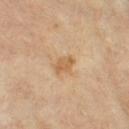Clinical impression: Part of a total-body skin-imaging series; this lesion was reviewed on a skin check and was not flagged for biopsy. Image and clinical context: Imaged with cross-polarized lighting. A 15 mm close-up extracted from a 3D total-body photography capture. The lesion's longest dimension is about 2.5 mm. The subject is a female roughly 75 years of age. The lesion is located on the left thigh.